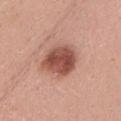{
  "biopsy_status": "not biopsied; imaged during a skin examination",
  "lesion_size": {
    "long_diameter_mm_approx": 4.5
  },
  "lighting": "white-light",
  "patient": {
    "sex": "female",
    "age_approx": 25
  },
  "image": {
    "source": "total-body photography crop",
    "field_of_view_mm": 15
  },
  "site": "head or neck"
}